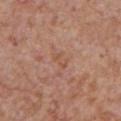<case>
<biopsy_status>not biopsied; imaged during a skin examination</biopsy_status>
<site>chest</site>
<image>
  <source>total-body photography crop</source>
  <field_of_view_mm>15</field_of_view_mm>
</image>
<patient>
  <sex>male</sex>
  <age_approx>65</age_approx>
</patient>
</case>